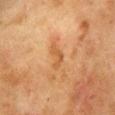Assessment:
Part of a total-body skin-imaging series; this lesion was reviewed on a skin check and was not flagged for biopsy.